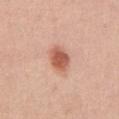Q: Was a biopsy performed?
A: total-body-photography surveillance lesion; no biopsy
Q: Lesion location?
A: the abdomen
Q: Who is the patient?
A: female, in their mid- to late 30s
Q: What kind of image is this?
A: 15 mm crop, total-body photography
Q: Lesion size?
A: about 3 mm
Q: What lighting was used for the tile?
A: white-light illumination